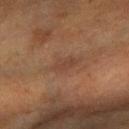diameter: about 3.5 mm | subject: female, in their mid-50s | illumination: cross-polarized | TBP lesion metrics: an average lesion color of about L≈34 a*≈16 b*≈24 (CIELAB), roughly 5 lightness units darker than nearby skin, and a normalized lesion–skin contrast near 5; a detector confidence of about 85 out of 100 that the crop contains a lesion | site: the arm | imaging modality: ~15 mm tile from a whole-body skin photo.